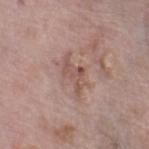| feature | finding |
|---|---|
| follow-up | total-body-photography surveillance lesion; no biopsy |
| anatomic site | the right thigh |
| acquisition | 15 mm crop, total-body photography |
| subject | female, in their 70s |